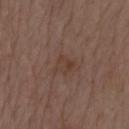Captured during whole-body skin photography for melanoma surveillance; the lesion was not biopsied.
A male patient approximately 70 years of age.
From the mid back.
A roughly 15 mm field-of-view crop from a total-body skin photograph.
Automated image analysis of the tile measured a border-irregularity rating of about 2.5/10, a color-variation rating of about 3/10, and a peripheral color-asymmetry measure near 1. It also reported an automated nevus-likeness rating near 0 out of 100 and a detector confidence of about 100 out of 100 that the crop contains a lesion.
About 2.5 mm across.